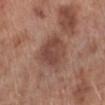Part of a total-body skin-imaging series; this lesion was reviewed on a skin check and was not flagged for biopsy.